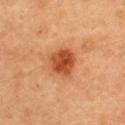This lesion was catalogued during total-body skin photography and was not selected for biopsy. About 3.5 mm across. A lesion tile, about 15 mm wide, cut from a 3D total-body photograph. An algorithmic analysis of the crop reported an eccentricity of roughly 0.4 and two-axis asymmetry of about 0.15. It also reported a lesion color around L≈44 a*≈29 b*≈37 in CIELAB, about 13 CIELAB-L* units darker than the surrounding skin, and a normalized border contrast of about 10. And it measured a border-irregularity rating of about 1.5/10 and a color-variation rating of about 4/10. Imaged with cross-polarized lighting. The lesion is located on the upper back. The subject is a female aged approximately 60.